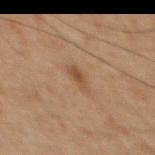diameter = ~2.5 mm (longest diameter)
site = the chest
lighting = cross-polarized
image source = total-body-photography crop, ~15 mm field of view
patient = male, roughly 70 years of age
automated lesion analysis = a border-irregularity index near 2.5/10 and peripheral color asymmetry of about 0.5